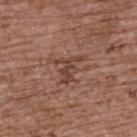Q: Was a biopsy performed?
A: total-body-photography surveillance lesion; no biopsy
Q: What is the anatomic site?
A: the upper back
Q: Patient demographics?
A: female, aged around 65
Q: What kind of image is this?
A: total-body-photography crop, ~15 mm field of view